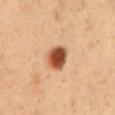No biopsy was performed on this lesion — it was imaged during a full skin examination and was not determined to be concerning. A male patient, about 50 years old. A region of skin cropped from a whole-body photographic capture, roughly 15 mm wide. Measured at roughly 3.5 mm in maximum diameter. Captured under cross-polarized illumination. On the chest.